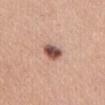<case>
<biopsy_status>not biopsied; imaged during a skin examination</biopsy_status>
<image>
  <source>total-body photography crop</source>
  <field_of_view_mm>15</field_of_view_mm>
</image>
<site>abdomen</site>
<automated_metrics>
  <area_mm2_approx>5.0</area_mm2_approx>
  <shape_asymmetry>0.2</shape_asymmetry>
  <cielab_L>51</cielab_L>
  <cielab_a>22</cielab_a>
  <cielab_b>26</cielab_b>
  <vs_skin_darker_L>19.0</vs_skin_darker_L>
  <vs_skin_contrast_norm>12.0</vs_skin_contrast_norm>
  <nevus_likeness_0_100>95</nevus_likeness_0_100>
  <lesion_detection_confidence_0_100>100</lesion_detection_confidence_0_100>
</automated_metrics>
<lesion_size>
  <long_diameter_mm_approx>3.0</long_diameter_mm_approx>
</lesion_size>
<patient>
  <sex>female</sex>
  <age_approx>35</age_approx>
</patient>
<lighting>white-light</lighting>
</case>